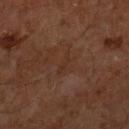Notes:
• biopsy status — no biopsy performed (imaged during a skin exam)
• location — the right lower leg
• diameter — about 2.5 mm
• imaging modality — 15 mm crop, total-body photography
• illumination — cross-polarized illumination
• patient — male, in their 60s